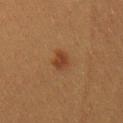* notes · no biopsy performed (imaged during a skin exam)
* body site · the right thigh
* illumination · cross-polarized illumination
* image source · ~15 mm tile from a whole-body skin photo
* lesion size · ≈2.5 mm
* TBP lesion metrics · about 8 CIELAB-L* units darker than the surrounding skin and a normalized lesion–skin contrast near 7.5
* subject · female, approximately 40 years of age